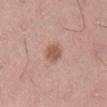The lesion was photographed on a routine skin check and not biopsied; there is no pathology result. A close-up tile cropped from a whole-body skin photograph, about 15 mm across. A female subject, aged around 40. About 3 mm across. From the right thigh.